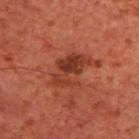{
  "lighting": "cross-polarized",
  "site": "upper back",
  "automated_metrics": {
    "border_irregularity_0_10": 6.5,
    "color_variation_0_10": 5.0,
    "nevus_likeness_0_100": 20
  },
  "patient": {
    "sex": "male",
    "age_approx": 70
  },
  "lesion_size": {
    "long_diameter_mm_approx": 4.5
  },
  "image": {
    "source": "total-body photography crop",
    "field_of_view_mm": 15
  }
}